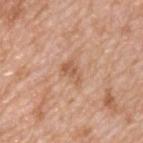Impression:
Imaged during a routine full-body skin examination; the lesion was not biopsied and no histopathology is available.
Context:
The lesion-visualizer software estimated a color-variation rating of about 2.5/10 and radial color variation of about 0.5. It also reported an automated nevus-likeness rating near 0 out of 100 and lesion-presence confidence of about 100/100. A roughly 15 mm field-of-view crop from a total-body skin photograph. A male patient, in their 50s. This is a white-light tile. From the chest.